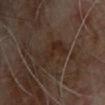Imaged during a routine full-body skin examination; the lesion was not biopsied and no histopathology is available. The tile uses cross-polarized illumination. Automated tile analysis of the lesion measured an area of roughly 8.5 mm² and an outline eccentricity of about 0.85 (0 = round, 1 = elongated). From the back. A region of skin cropped from a whole-body photographic capture, roughly 15 mm wide. A male patient, in their mid- to late 60s.